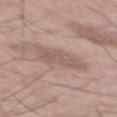follow-up = catalogued during a skin exam; not biopsied
anatomic site = the mid back
patient = male, about 55 years old
acquisition = total-body-photography crop, ~15 mm field of view
lesion size = ~5.5 mm (longest diameter)